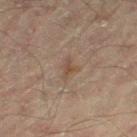Part of a total-body skin-imaging series; this lesion was reviewed on a skin check and was not flagged for biopsy. A male subject, aged 43 to 47. A roughly 15 mm field-of-view crop from a total-body skin photograph. Located on the left thigh.